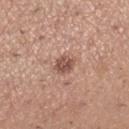Case summary:
* notes: total-body-photography surveillance lesion; no biopsy
* patient: female, in their 30s
* image-analysis metrics: a footprint of about 4.5 mm², an eccentricity of roughly 0.6, and two-axis asymmetry of about 0.15; border irregularity of about 1.5 on a 0–10 scale, a within-lesion color-variation index near 3.5/10, and a peripheral color-asymmetry measure near 1; a nevus-likeness score of about 85/100 and a lesion-detection confidence of about 100/100
* image: 15 mm crop, total-body photography
* tile lighting: white-light illumination
* diameter: ≈3 mm
* location: the right lower leg